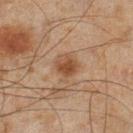Recorded during total-body skin imaging; not selected for excision or biopsy. An algorithmic analysis of the crop reported an automated nevus-likeness rating near 80 out of 100 and a lesion-detection confidence of about 100/100. A male patient, about 45 years old. Measured at roughly 3 mm in maximum diameter. A close-up tile cropped from a whole-body skin photograph, about 15 mm across. From the leg. The tile uses cross-polarized illumination.